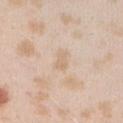{
  "biopsy_status": "not biopsied; imaged during a skin examination",
  "site": "arm",
  "patient": {
    "sex": "female",
    "age_approx": 25
  },
  "lighting": "white-light",
  "lesion_size": {
    "long_diameter_mm_approx": 2.5
  },
  "image": {
    "source": "total-body photography crop",
    "field_of_view_mm": 15
  }
}